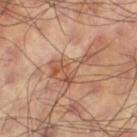workup: no biopsy performed (imaged during a skin exam); site: the leg; image: 15 mm crop, total-body photography; subject: male, aged approximately 60; tile lighting: cross-polarized.